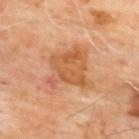biopsy status — catalogued during a skin exam; not biopsied | lesion size — ≈5.5 mm | acquisition — ~15 mm tile from a whole-body skin photo | patient — male, in their mid- to late 60s | anatomic site — the upper back | lighting — cross-polarized.